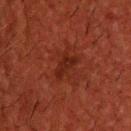From the upper back. The patient is a male about 50 years old. Imaged with cross-polarized lighting. Measured at roughly 3.5 mm in maximum diameter. A close-up tile cropped from a whole-body skin photograph, about 15 mm across.